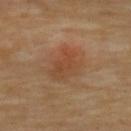Assessment: This lesion was catalogued during total-body skin photography and was not selected for biopsy. Clinical summary: A female patient, approximately 70 years of age. From the upper back. A 15 mm close-up tile from a total-body photography series done for melanoma screening. Imaged with cross-polarized lighting.